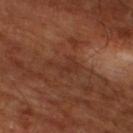Impression: The lesion was tiled from a total-body skin photograph and was not biopsied. Background: The lesion is on the left lower leg. A 15 mm close-up extracted from a 3D total-body photography capture. A patient approximately 65 years of age.